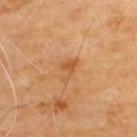An algorithmic analysis of the crop reported an outline eccentricity of about 0.75 (0 = round, 1 = elongated) and a shape-asymmetry score of about 0.55 (0 = symmetric). It also reported a lesion color around L≈54 a*≈24 b*≈41 in CIELAB. The analysis additionally found a border-irregularity rating of about 6.5/10 and internal color variation of about 0.5 on a 0–10 scale. It also reported an automated nevus-likeness rating near 10 out of 100. Captured under cross-polarized illumination. Located on the upper back. A male patient aged 68 to 72. The recorded lesion diameter is about 3 mm. A close-up tile cropped from a whole-body skin photograph, about 15 mm across.